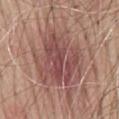follow-up: no biopsy performed (imaged during a skin exam)
anatomic site: the mid back
lighting: white-light illumination
image source: 15 mm crop, total-body photography
lesion size: ~13 mm (longest diameter)
subject: male, aged approximately 65
automated lesion analysis: a lesion color around L≈50 a*≈22 b*≈23 in CIELAB, roughly 11 lightness units darker than nearby skin, and a normalized border contrast of about 8; a nevus-likeness score of about 0/100 and a detector confidence of about 100 out of 100 that the crop contains a lesion